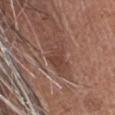biopsy_status: not biopsied; imaged during a skin examination
patient:
  sex: male
  age_approx: 60
lesion_size:
  long_diameter_mm_approx: 4.5
lighting: white-light
site: head or neck
image:
  source: total-body photography crop
  field_of_view_mm: 15